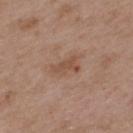{
  "biopsy_status": "not biopsied; imaged during a skin examination",
  "image": {
    "source": "total-body photography crop",
    "field_of_view_mm": 15
  },
  "patient": {
    "sex": "male",
    "age_approx": 50
  },
  "lesion_size": {
    "long_diameter_mm_approx": 3.5
  },
  "site": "back",
  "lighting": "white-light"
}